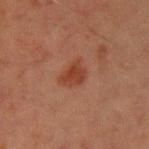<case>
  <biopsy_status>not biopsied; imaged during a skin examination</biopsy_status>
  <site>left upper arm</site>
  <patient>
    <sex>male</sex>
    <age_approx>45</age_approx>
  </patient>
  <lighting>cross-polarized</lighting>
  <image>
    <source>total-body photography crop</source>
    <field_of_view_mm>15</field_of_view_mm>
  </image>
  <lesion_size>
    <long_diameter_mm_approx>3.0</long_diameter_mm_approx>
  </lesion_size>
</case>